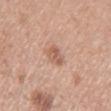follow-up — total-body-photography surveillance lesion; no biopsy | subject — female, about 60 years old | location — the left upper arm | image — ~15 mm crop, total-body skin-cancer survey | diameter — ≈2.5 mm | lighting — white-light illumination.